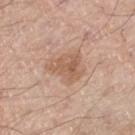Assessment:
Recorded during total-body skin imaging; not selected for excision or biopsy.
Context:
Measured at roughly 4 mm in maximum diameter. The patient is a male in their 70s. Imaged with white-light lighting. The lesion is on the right thigh. A roughly 15 mm field-of-view crop from a total-body skin photograph.